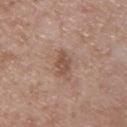biopsy status: catalogued during a skin exam; not biopsied | location: the right upper arm | image source: ~15 mm crop, total-body skin-cancer survey | patient: male, aged 48 to 52 | lesion diameter: ≈2.5 mm | lighting: white-light illumination.